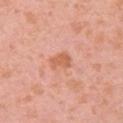Clinical impression:
The lesion was tiled from a total-body skin photograph and was not biopsied.
Image and clinical context:
The subject is a female approximately 40 years of age. Approximately 2.5 mm at its widest. The lesion is located on the chest. Captured under white-light illumination. A close-up tile cropped from a whole-body skin photograph, about 15 mm across.